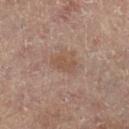Q: Who is the patient?
A: female, aged 78–82
Q: What is the imaging modality?
A: ~15 mm crop, total-body skin-cancer survey
Q: Where on the body is the lesion?
A: the right leg